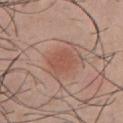workup — total-body-photography surveillance lesion; no biopsy | patient — male, aged around 30 | lesion size — about 3 mm | lighting — white-light illumination | body site — the chest | image — total-body-photography crop, ~15 mm field of view.